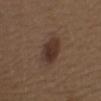Background:
Longest diameter approximately 4 mm. The subject is a female aged 38 to 42. A 15 mm close-up extracted from a 3D total-body photography capture. Imaged with white-light lighting. From the upper back.